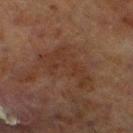– notes — catalogued during a skin exam; not biopsied
– lesion size — ~6.5 mm (longest diameter)
– imaging modality — ~15 mm tile from a whole-body skin photo
– site — the left lower leg
– lighting — cross-polarized
– image-analysis metrics — an area of roughly 15 mm² and a symmetry-axis asymmetry near 0.55
– subject — male, about 70 years old